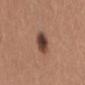Q: Is there a histopathology result?
A: imaged on a skin check; not biopsied
Q: Where on the body is the lesion?
A: the lower back
Q: What lighting was used for the tile?
A: white-light
Q: Automated lesion metrics?
A: a lesion area of about 6.5 mm² and a symmetry-axis asymmetry near 0.25; a lesion–skin lightness drop of about 15; a border-irregularity rating of about 2/10, a color-variation rating of about 5.5/10, and radial color variation of about 1.5; an automated nevus-likeness rating near 75 out of 100 and a lesion-detection confidence of about 100/100
Q: Patient demographics?
A: female, in their mid-50s
Q: What kind of image is this?
A: ~15 mm tile from a whole-body skin photo
Q: What is the lesion's diameter?
A: ~3 mm (longest diameter)